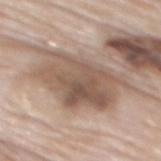<tbp_lesion>
  <biopsy_status>not biopsied; imaged during a skin examination</biopsy_status>
  <lighting>white-light</lighting>
  <automated_metrics>
    <cielab_L>55</cielab_L>
    <cielab_a>15</cielab_a>
    <cielab_b>26</cielab_b>
    <vs_skin_darker_L>13.0</vs_skin_darker_L>
    <vs_skin_contrast_norm>8.5</vs_skin_contrast_norm>
    <nevus_likeness_0_100>5</nevus_likeness_0_100>
  </automated_metrics>
  <lesion_size>
    <long_diameter_mm_approx>10.0</long_diameter_mm_approx>
  </lesion_size>
  <site>back</site>
  <patient>
    <sex>male</sex>
    <age_approx>85+</age_approx>
  </patient>
  <image>
    <source>total-body photography crop</source>
    <field_of_view_mm>15</field_of_view_mm>
  </image>
</tbp_lesion>